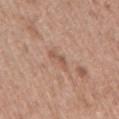notes — no biopsy performed (imaged during a skin exam) | illumination — white-light | lesion size — about 3 mm | imaging modality — 15 mm crop, total-body photography | patient — female, aged 58 to 62 | TBP lesion metrics — a border-irregularity rating of about 6/10 and radial color variation of about 0; lesion-presence confidence of about 100/100 | location — the right upper arm.